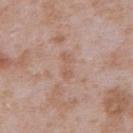Assessment: Recorded during total-body skin imaging; not selected for excision or biopsy. Context: The lesion is on the abdomen. Cropped from a whole-body photographic skin survey; the tile spans about 15 mm. The subject is a male about 65 years old.